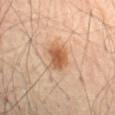Assessment:
This lesion was catalogued during total-body skin photography and was not selected for biopsy.
Image and clinical context:
This is a cross-polarized tile. A roughly 15 mm field-of-view crop from a total-body skin photograph. The patient is a male in their 70s. Located on the front of the torso. An algorithmic analysis of the crop reported a footprint of about 8 mm², a shape eccentricity near 0.7, and a shape-asymmetry score of about 0.15 (0 = symmetric). The software also gave an average lesion color of about L≈57 a*≈22 b*≈36 (CIELAB), a lesion–skin lightness drop of about 12, and a lesion-to-skin contrast of about 8.5 (normalized; higher = more distinct). It also reported internal color variation of about 4.5 on a 0–10 scale and a peripheral color-asymmetry measure near 1.5. The recorded lesion diameter is about 4 mm.